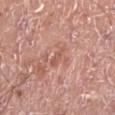biopsy status: imaged on a skin check; not biopsied
body site: the left lower leg
acquisition: ~15 mm crop, total-body skin-cancer survey
lighting: white-light illumination
patient: male, aged 18–22